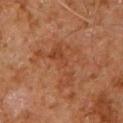Part of a total-body skin-imaging series; this lesion was reviewed on a skin check and was not flagged for biopsy.
The subject is a male in their 60s.
On the upper back.
This image is a 15 mm lesion crop taken from a total-body photograph.